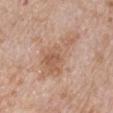No biopsy was performed on this lesion — it was imaged during a full skin examination and was not determined to be concerning. Automated tile analysis of the lesion measured a lesion color around L≈59 a*≈19 b*≈30 in CIELAB. And it measured a border-irregularity rating of about 6/10, internal color variation of about 4 on a 0–10 scale, and a peripheral color-asymmetry measure near 1. Imaged with white-light lighting. The patient is a male aged around 70. The lesion is located on the right upper arm. A close-up tile cropped from a whole-body skin photograph, about 15 mm across.